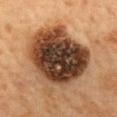patient:
  sex: female
  age_approx: 60
image:
  source: total-body photography crop
  field_of_view_mm: 15
lesion_size:
  long_diameter_mm_approx: 8.5
site: mid back